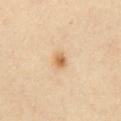follow-up — imaged on a skin check; not biopsied | acquisition — ~15 mm tile from a whole-body skin photo | site — the chest | automated metrics — a lesion color around L≈66 a*≈20 b*≈41 in CIELAB and roughly 11 lightness units darker than nearby skin; a within-lesion color-variation index near 3/10 and radial color variation of about 1 | subject — female, aged 38–42 | illumination — cross-polarized illumination | lesion diameter — about 2 mm.